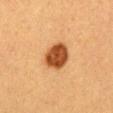{"biopsy_status": "not biopsied; imaged during a skin examination", "patient": {"sex": "female", "age_approx": 40}, "site": "chest", "lesion_size": {"long_diameter_mm_approx": 4.0}, "image": {"source": "total-body photography crop", "field_of_view_mm": 15}, "lighting": "cross-polarized", "automated_metrics": {"area_mm2_approx": 10.0, "eccentricity": 0.6, "shape_asymmetry": 0.15}}